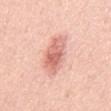Part of a total-body skin-imaging series; this lesion was reviewed on a skin check and was not flagged for biopsy. A male subject in their mid- to late 50s. Cropped from a whole-body photographic skin survey; the tile spans about 15 mm. Located on the chest. Longest diameter approximately 6 mm. Automated image analysis of the tile measured an outline eccentricity of about 0.9 (0 = round, 1 = elongated) and a symmetry-axis asymmetry near 0.2. And it measured a mean CIELAB color near L≈68 a*≈27 b*≈28 and about 13 CIELAB-L* units darker than the surrounding skin. The tile uses white-light illumination.